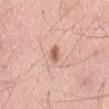<lesion>
  <biopsy_status>not biopsied; imaged during a skin examination</biopsy_status>
  <lesion_size>
    <long_diameter_mm_approx>2.5</long_diameter_mm_approx>
  </lesion_size>
  <lighting>white-light</lighting>
  <patient>
    <sex>male</sex>
    <age_approx>60</age_approx>
  </patient>
  <image>
    <source>total-body photography crop</source>
    <field_of_view_mm>15</field_of_view_mm>
  </image>
  <automated_metrics>
    <area_mm2_approx>3.0</area_mm2_approx>
    <shape_asymmetry>0.3</shape_asymmetry>
    <cielab_L>62</cielab_L>
    <cielab_a>23</cielab_a>
    <cielab_b>29</cielab_b>
    <vs_skin_darker_L>12.0</vs_skin_darker_L>
    <vs_skin_contrast_norm>7.5</vs_skin_contrast_norm>
    <border_irregularity_0_10>3.0</border_irregularity_0_10>
    <peripheral_color_asymmetry>1.0</peripheral_color_asymmetry>
    <nevus_likeness_0_100>80</nevus_likeness_0_100>
  </automated_metrics>
  <site>mid back</site>
</lesion>